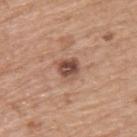The lesion was photographed on a routine skin check and not biopsied; there is no pathology result.
From the back.
A roughly 15 mm field-of-view crop from a total-body skin photograph.
The subject is a male aged around 75.